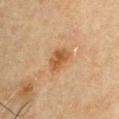notes: total-body-photography surveillance lesion; no biopsy
image-analysis metrics: an area of roughly 5.5 mm² and a symmetry-axis asymmetry near 0.2; an average lesion color of about L≈44 a*≈18 b*≈34 (CIELAB) and roughly 9 lightness units darker than nearby skin; a classifier nevus-likeness of about 80/100 and a detector confidence of about 100 out of 100 that the crop contains a lesion
illumination: cross-polarized
acquisition: total-body-photography crop, ~15 mm field of view
anatomic site: the right upper arm
subject: male, approximately 70 years of age